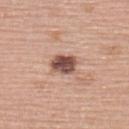Assessment: Recorded during total-body skin imaging; not selected for excision or biopsy. Acquisition and patient details: A 15 mm crop from a total-body photograph taken for skin-cancer surveillance. The subject is a female approximately 60 years of age. Located on the upper back.